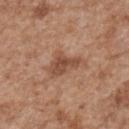Impression:
Imaged during a routine full-body skin examination; the lesion was not biopsied and no histopathology is available.
Acquisition and patient details:
The tile uses white-light illumination. The lesion is on the back. A 15 mm close-up tile from a total-body photography series done for melanoma screening. Longest diameter approximately 4.5 mm. The subject is a male about 65 years old.